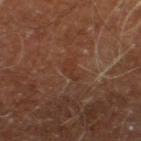Findings:
– workup — catalogued during a skin exam; not biopsied
– anatomic site — the right leg
– image — total-body-photography crop, ~15 mm field of view
– subject — male, aged 58–62
– tile lighting — cross-polarized illumination
– lesion diameter — ~2.5 mm (longest diameter)
– image-analysis metrics — a detector confidence of about 85 out of 100 that the crop contains a lesion